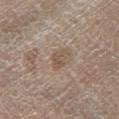Assessment: No biopsy was performed on this lesion — it was imaged during a full skin examination and was not determined to be concerning.